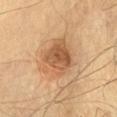biopsy status: imaged on a skin check; not biopsied | lesion diameter: ≈5.5 mm | subject: male, aged 63–67 | image source: 15 mm crop, total-body photography | illumination: cross-polarized.